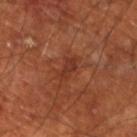  biopsy_status: not biopsied; imaged during a skin examination
  lesion_size:
    long_diameter_mm_approx: 3.0
  site: left lower leg
  patient:
    sex: male
    age_approx: 70
  image:
    source: total-body photography crop
    field_of_view_mm: 15